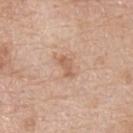Captured during whole-body skin photography for melanoma surveillance; the lesion was not biopsied.